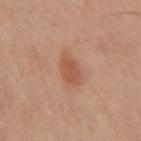Findings:
• notes — no biopsy performed (imaged during a skin exam)
• subject — male, in their mid-40s
• tile lighting — cross-polarized
• body site — the mid back
• lesion size — ~3.5 mm (longest diameter)
• image — 15 mm crop, total-body photography